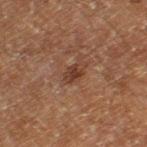<tbp_lesion>
<lighting>cross-polarized</lighting>
<automated_metrics>
  <shape_asymmetry>0.15</shape_asymmetry>
  <cielab_L>28</cielab_L>
  <cielab_a>17</cielab_a>
  <cielab_b>23</cielab_b>
  <nevus_likeness_0_100>30</nevus_likeness_0_100>
  <lesion_detection_confidence_0_100>100</lesion_detection_confidence_0_100>
</automated_metrics>
<image>
  <source>total-body photography crop</source>
  <field_of_view_mm>15</field_of_view_mm>
</image>
<lesion_size>
  <long_diameter_mm_approx>2.5</long_diameter_mm_approx>
</lesion_size>
<patient>
  <sex>male</sex>
  <age_approx>60</age_approx>
</patient>
<site>left thigh</site>
</tbp_lesion>